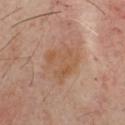The lesion was tiled from a total-body skin photograph and was not biopsied.
Imaged with cross-polarized lighting.
Measured at roughly 4.5 mm in maximum diameter.
The subject is about 55 years old.
Located on the chest.
A 15 mm close-up tile from a total-body photography series done for melanoma screening.